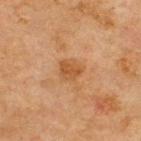Clinical summary: Approximately 2.5 mm at its widest. Located on the upper back. Cropped from a whole-body photographic skin survey; the tile spans about 15 mm. The tile uses cross-polarized illumination. A male subject aged 68–72.